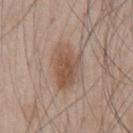Impression:
Recorded during total-body skin imaging; not selected for excision or biopsy.
Background:
The patient is a male about 45 years old. Automated image analysis of the tile measured an area of roughly 14 mm², an eccentricity of roughly 0.75, and a symmetry-axis asymmetry near 0.25. And it measured an average lesion color of about L≈52 a*≈18 b*≈28 (CIELAB), about 9 CIELAB-L* units darker than the surrounding skin, and a normalized lesion–skin contrast near 7. The software also gave internal color variation of about 4.5 on a 0–10 scale and radial color variation of about 1.5. Captured under white-light illumination. The lesion is located on the chest. The lesion's longest dimension is about 5 mm. A close-up tile cropped from a whole-body skin photograph, about 15 mm across.